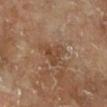Impression: Imaged during a routine full-body skin examination; the lesion was not biopsied and no histopathology is available. Acquisition and patient details: The lesion is on the right lower leg. A 15 mm close-up tile from a total-body photography series done for melanoma screening. A male subject aged around 65.